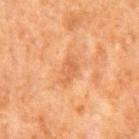Q: Lesion size?
A: ≈3.5 mm
Q: Who is the patient?
A: male, roughly 65 years of age
Q: How was this image acquired?
A: total-body-photography crop, ~15 mm field of view
Q: Lesion location?
A: the back